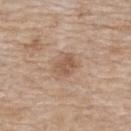Impression:
This lesion was catalogued during total-body skin photography and was not selected for biopsy.
Context:
Cropped from a total-body skin-imaging series; the visible field is about 15 mm. A female patient, in their mid-70s. The lesion is located on the upper back.